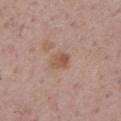{
  "image": {
    "source": "total-body photography crop",
    "field_of_view_mm": 15
  },
  "lesion_size": {
    "long_diameter_mm_approx": 2.5
  },
  "site": "front of the torso",
  "patient": {
    "sex": "male",
    "age_approx": 75
  }
}